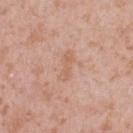No biopsy was performed on this lesion — it was imaged during a full skin examination and was not determined to be concerning.
The lesion is on the back.
Automated tile analysis of the lesion measured a footprint of about 4.5 mm², an eccentricity of roughly 0.9, and a shape-asymmetry score of about 0.35 (0 = symmetric). The analysis additionally found a lesion color around L≈62 a*≈21 b*≈30 in CIELAB, about 6 CIELAB-L* units darker than the surrounding skin, and a normalized border contrast of about 5. The analysis additionally found border irregularity of about 4 on a 0–10 scale, a within-lesion color-variation index near 1/10, and radial color variation of about 0.
A region of skin cropped from a whole-body photographic capture, roughly 15 mm wide.
A female subject aged 38 to 42.
Captured under white-light illumination.